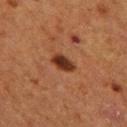<case>
<biopsy_status>not biopsied; imaged during a skin examination</biopsy_status>
<automated_metrics>
  <area_mm2_approx>5.0</area_mm2_approx>
  <eccentricity>0.8</eccentricity>
  <shape_asymmetry>0.2</shape_asymmetry>
  <vs_skin_contrast_norm>12.0</vs_skin_contrast_norm>
  <color_variation_0_10>3.5</color_variation_0_10>
  <peripheral_color_asymmetry>1.0</peripheral_color_asymmetry>
</automated_metrics>
<patient>
  <sex>female</sex>
  <age_approx>50</age_approx>
</patient>
<lighting>cross-polarized</lighting>
<image>
  <source>total-body photography crop</source>
  <field_of_view_mm>15</field_of_view_mm>
</image>
<site>leg</site>
</case>